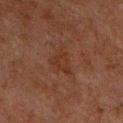Impression:
Recorded during total-body skin imaging; not selected for excision or biopsy.
Background:
The lesion is on the chest. A male patient aged 58 to 62. A close-up tile cropped from a whole-body skin photograph, about 15 mm across. About 4 mm across. Captured under cross-polarized illumination. An algorithmic analysis of the crop reported a footprint of about 7.5 mm² and a symmetry-axis asymmetry near 0.45. The analysis additionally found a border-irregularity index near 4.5/10. The software also gave an automated nevus-likeness rating near 0 out of 100.